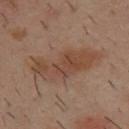A 15 mm close-up extracted from a 3D total-body photography capture. The lesion is on the upper back. A male patient aged approximately 40.